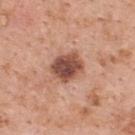workup = catalogued during a skin exam; not biopsied | TBP lesion metrics = a lesion color around L≈50 a*≈23 b*≈28 in CIELAB, roughly 16 lightness units darker than nearby skin, and a normalized border contrast of about 10.5; a border-irregularity rating of about 1.5/10, a within-lesion color-variation index near 5.5/10, and radial color variation of about 1.5; an automated nevus-likeness rating near 35 out of 100 | acquisition = ~15 mm tile from a whole-body skin photo | subject = female, aged approximately 50 | lesion diameter = ~4 mm (longest diameter) | location = the upper back | lighting = white-light.